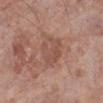| key | value |
|---|---|
| notes | catalogued during a skin exam; not biopsied |
| body site | the leg |
| patient | female, aged around 75 |
| image | total-body-photography crop, ~15 mm field of view |